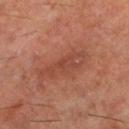Impression: This lesion was catalogued during total-body skin photography and was not selected for biopsy. Image and clinical context: Imaged with cross-polarized lighting. A male patient aged around 60. Approximately 5.5 mm at its widest. A close-up tile cropped from a whole-body skin photograph, about 15 mm across. Automated image analysis of the tile measured a lesion color around L≈42 a*≈25 b*≈28 in CIELAB, roughly 7 lightness units darker than nearby skin, and a normalized lesion–skin contrast near 5.5. And it measured an automated nevus-likeness rating near 0 out of 100. From the left lower leg.